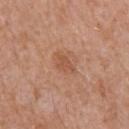Captured during whole-body skin photography for melanoma surveillance; the lesion was not biopsied. A 15 mm crop from a total-body photograph taken for skin-cancer surveillance. Imaged with white-light lighting. Measured at roughly 3 mm in maximum diameter. The lesion is on the back. A male subject about 60 years old. An algorithmic analysis of the crop reported an automated nevus-likeness rating near 30 out of 100 and a lesion-detection confidence of about 100/100.